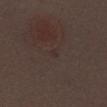Recorded during total-body skin imaging; not selected for excision or biopsy.